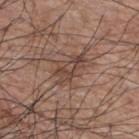{
  "biopsy_status": "not biopsied; imaged during a skin examination",
  "patient": {
    "sex": "male",
    "age_approx": 70
  },
  "image": {
    "source": "total-body photography crop",
    "field_of_view_mm": 15
  },
  "site": "upper back"
}